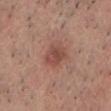Imaged during a routine full-body skin examination; the lesion was not biopsied and no histopathology is available.
A 15 mm crop from a total-body photograph taken for skin-cancer surveillance.
Automated tile analysis of the lesion measured a mean CIELAB color near L≈48 a*≈23 b*≈26 and a normalized lesion–skin contrast near 6.5. And it measured a border-irregularity rating of about 2/10 and radial color variation of about 1.5. The analysis additionally found a classifier nevus-likeness of about 50/100 and a lesion-detection confidence of about 100/100.
The tile uses white-light illumination.
From the chest.
The subject is a male approximately 45 years of age.